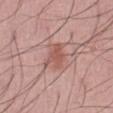{
  "biopsy_status": "not biopsied; imaged during a skin examination",
  "patient": {
    "sex": "male",
    "age_approx": 50
  },
  "lighting": "white-light",
  "image": {
    "source": "total-body photography crop",
    "field_of_view_mm": 15
  },
  "site": "abdomen",
  "lesion_size": {
    "long_diameter_mm_approx": 3.5
  }
}